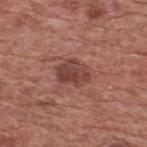notes = catalogued during a skin exam; not biopsied
location = the upper back
image source = ~15 mm tile from a whole-body skin photo
patient = male, aged 73 to 77